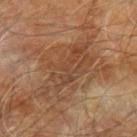Imaged during a routine full-body skin examination; the lesion was not biopsied and no histopathology is available. A 15 mm close-up tile from a total-body photography series done for melanoma screening. This is a cross-polarized tile. Measured at roughly 8 mm in maximum diameter. The lesion is located on the left forearm. The total-body-photography lesion software estimated a footprint of about 19 mm², an outline eccentricity of about 0.9 (0 = round, 1 = elongated), and a shape-asymmetry score of about 0.35 (0 = symmetric). And it measured an automated nevus-likeness rating near 0 out of 100 and a lesion-detection confidence of about 85/100. A male patient, roughly 70 years of age.